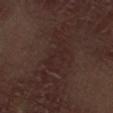<case>
  <biopsy_status>not biopsied; imaged during a skin examination</biopsy_status>
  <patient>
    <sex>male</sex>
    <age_approx>70</age_approx>
  </patient>
  <lesion_size>
    <long_diameter_mm_approx>4.5</long_diameter_mm_approx>
  </lesion_size>
  <automated_metrics>
    <vs_skin_darker_L>4.0</vs_skin_darker_L>
    <vs_skin_contrast_norm>5.0</vs_skin_contrast_norm>
    <nevus_likeness_0_100>0</nevus_likeness_0_100>
    <lesion_detection_confidence_0_100>50</lesion_detection_confidence_0_100>
  </automated_metrics>
  <image>
    <source>total-body photography crop</source>
    <field_of_view_mm>15</field_of_view_mm>
  </image>
  <site>leg</site>
  <lighting>white-light</lighting>
</case>